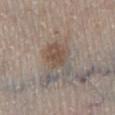Case summary:
– follow-up — no biopsy performed (imaged during a skin exam)
– lesion diameter — ~5.5 mm (longest diameter)
– anatomic site — the leg
– image — total-body-photography crop, ~15 mm field of view
– illumination — white-light illumination
– subject — male, in their 60s